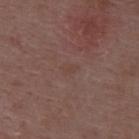Assessment:
The lesion was tiled from a total-body skin photograph and was not biopsied.
Clinical summary:
A 15 mm close-up tile from a total-body photography series done for melanoma screening. Automated image analysis of the tile measured roughly 3 lightness units darker than nearby skin and a lesion-to-skin contrast of about 3.5 (normalized; higher = more distinct). Captured under white-light illumination. Measured at roughly 2 mm in maximum diameter. The patient is a female roughly 45 years of age. The lesion is located on the back.